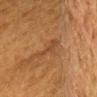  lesion_size:
    long_diameter_mm_approx: 4.0
  site: head or neck
  image:
    source: total-body photography crop
    field_of_view_mm: 15
  patient:
    sex: male
    age_approx: 50